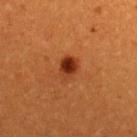Case summary:
– workup: imaged on a skin check; not biopsied
– anatomic site: the back
– image: ~15 mm crop, total-body skin-cancer survey
– subject: female, in their 30s